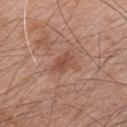tile lighting: white-light illumination; site: the chest; patient: male, in their mid-40s; acquisition: 15 mm crop, total-body photography; diameter: ≈3.5 mm.